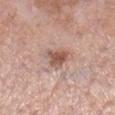Case summary:
- site · the right lower leg
- imaging modality · total-body-photography crop, ~15 mm field of view
- patient · female, in their mid- to late 50s